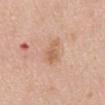Impression: Imaged during a routine full-body skin examination; the lesion was not biopsied and no histopathology is available. Context: Approximately 3.5 mm at its widest. This image is a 15 mm lesion crop taken from a total-body photograph. The tile uses white-light illumination. A male subject roughly 70 years of age. An algorithmic analysis of the crop reported a footprint of about 5 mm², an outline eccentricity of about 0.85 (0 = round, 1 = elongated), and a shape-asymmetry score of about 0.3 (0 = symmetric). On the abdomen.